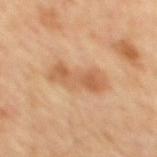Case summary:
- workup · no biopsy performed (imaged during a skin exam)
- tile lighting · cross-polarized
- location · the mid back
- TBP lesion metrics · roughly 9 lightness units darker than nearby skin and a lesion-to-skin contrast of about 6 (normalized; higher = more distinct); a border-irregularity index near 3.5/10 and a within-lesion color-variation index near 4/10
- diameter · ≈6 mm
- subject · male, aged 58 to 62
- imaging modality · 15 mm crop, total-body photography